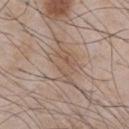follow-up: catalogued during a skin exam; not biopsied | illumination: white-light | location: the chest | diameter: ≈4.5 mm | TBP lesion metrics: a lesion area of about 8.5 mm², an eccentricity of roughly 0.75, and a symmetry-axis asymmetry near 0.45; an automated nevus-likeness rating near 0 out of 100 and lesion-presence confidence of about 90/100 | image: ~15 mm crop, total-body skin-cancer survey | patient: male, roughly 50 years of age.